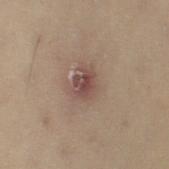<lesion>
<biopsy_status>not biopsied; imaged during a skin examination</biopsy_status>
<image>
  <source>total-body photography crop</source>
  <field_of_view_mm>15</field_of_view_mm>
</image>
<automated_metrics>
  <area_mm2_approx>8.0</area_mm2_approx>
  <eccentricity>0.65</eccentricity>
  <cielab_L>40</cielab_L>
  <cielab_a>15</cielab_a>
  <cielab_b>19</cielab_b>
  <vs_skin_darker_L>8.0</vs_skin_darker_L>
</automated_metrics>
<patient>
  <sex>female</sex>
  <age_approx>50</age_approx>
</patient>
<lighting>cross-polarized</lighting>
<site>right thigh</site>
<lesion_size>
  <long_diameter_mm_approx>3.5</long_diameter_mm_approx>
</lesion_size>
</lesion>